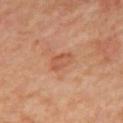The lesion was tiled from a total-body skin photograph and was not biopsied. The lesion's longest dimension is about 3 mm. A lesion tile, about 15 mm wide, cut from a 3D total-body photograph. Imaged with cross-polarized lighting. From the mid back. A male patient, roughly 60 years of age.